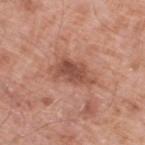| feature | finding |
|---|---|
| biopsy status | total-body-photography surveillance lesion; no biopsy |
| acquisition | total-body-photography crop, ~15 mm field of view |
| subject | male, about 80 years old |
| illumination | white-light |
| location | the left lower leg |
| TBP lesion metrics | a lesion area of about 8.5 mm² and a shape-asymmetry score of about 0.3 (0 = symmetric); a lesion color around L≈50 a*≈24 b*≈29 in CIELAB, a lesion–skin lightness drop of about 12, and a lesion-to-skin contrast of about 8 (normalized; higher = more distinct); an automated nevus-likeness rating near 20 out of 100 and a lesion-detection confidence of about 100/100 |